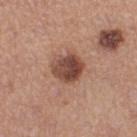Part of a total-body skin-imaging series; this lesion was reviewed on a skin check and was not flagged for biopsy.
Captured under white-light illumination.
Measured at roughly 3.5 mm in maximum diameter.
A lesion tile, about 15 mm wide, cut from a 3D total-body photograph.
A female subject, about 35 years old.
Located on the left thigh.